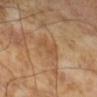Imaged during a routine full-body skin examination; the lesion was not biopsied and no histopathology is available. The recorded lesion diameter is about 3.5 mm. The patient is a male aged 63–67. A roughly 15 mm field-of-view crop from a total-body skin photograph. Imaged with cross-polarized lighting.